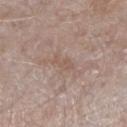Notes:
- patient — male, aged approximately 45
- acquisition — ~15 mm crop, total-body skin-cancer survey
- location — the leg
- illumination — white-light illumination